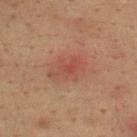The lesion's longest dimension is about 4.5 mm.
The patient is a male aged 33–37.
The lesion is located on the upper back.
A 15 mm crop from a total-body photograph taken for skin-cancer surveillance.
Imaged with cross-polarized lighting.
Automated tile analysis of the lesion measured border irregularity of about 2.5 on a 0–10 scale and a color-variation rating of about 4.5/10.